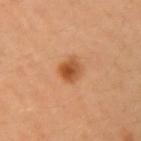  biopsy_status: not biopsied; imaged during a skin examination
  site: right upper arm
  lighting: cross-polarized
  image:
    source: total-body photography crop
    field_of_view_mm: 15
  automated_metrics:
    nevus_likeness_0_100: 95
    lesion_detection_confidence_0_100: 100
  patient:
    sex: male
    age_approx: 40